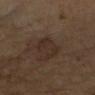• workup — catalogued during a skin exam; not biopsied
• imaging modality — ~15 mm tile from a whole-body skin photo
• TBP lesion metrics — an area of roughly 8 mm², an eccentricity of roughly 0.75, and two-axis asymmetry of about 0.3; a classifier nevus-likeness of about 15/100 and a detector confidence of about 100 out of 100 that the crop contains a lesion
• location — the left arm
• subject — male, about 65 years old